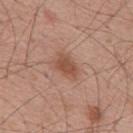Longest diameter approximately 4 mm.
The patient is a male approximately 55 years of age.
The lesion is located on the mid back.
A 15 mm close-up tile from a total-body photography series done for melanoma screening.